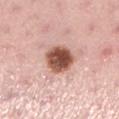{
  "biopsy_status": "not biopsied; imaged during a skin examination",
  "patient": {
    "sex": "female",
    "age_approx": 35
  },
  "image": {
    "source": "total-body photography crop",
    "field_of_view_mm": 15
  },
  "site": "left lower leg"
}